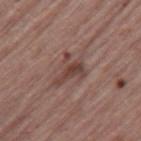| feature | finding |
|---|---|
| biopsy status | imaged on a skin check; not biopsied |
| image | ~15 mm crop, total-body skin-cancer survey |
| lighting | white-light |
| lesion diameter | about 4 mm |
| TBP lesion metrics | a nevus-likeness score of about 0/100 and a detector confidence of about 100 out of 100 that the crop contains a lesion |
| subject | male, roughly 65 years of age |
| location | the right thigh |